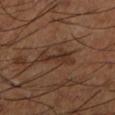Q: Is there a histopathology result?
A: total-body-photography surveillance lesion; no biopsy